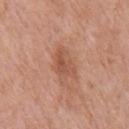Captured during whole-body skin photography for melanoma surveillance; the lesion was not biopsied. A region of skin cropped from a whole-body photographic capture, roughly 15 mm wide. A male subject approximately 70 years of age. From the chest. Captured under white-light illumination. Approximately 4 mm at its widest.